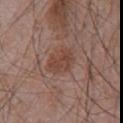Captured during whole-body skin photography for melanoma surveillance; the lesion was not biopsied. The lesion is on the chest. The lesion's longest dimension is about 3.5 mm. This image is a 15 mm lesion crop taken from a total-body photograph. Captured under white-light illumination. A male subject aged approximately 70.